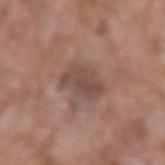follow-up — catalogued during a skin exam; not biopsied
image — ~15 mm crop, total-body skin-cancer survey
subject — male, roughly 60 years of age
body site — the left upper arm
lesion size — ≈4.5 mm
image-analysis metrics — an area of roughly 9.5 mm², an outline eccentricity of about 0.8 (0 = round, 1 = elongated), and two-axis asymmetry of about 0.2; an average lesion color of about L≈47 a*≈17 b*≈23 (CIELAB); border irregularity of about 2.5 on a 0–10 scale, internal color variation of about 3 on a 0–10 scale, and a peripheral color-asymmetry measure near 1; a classifier nevus-likeness of about 0/100 and a lesion-detection confidence of about 100/100